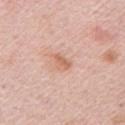| key | value |
|---|---|
| notes | total-body-photography surveillance lesion; no biopsy |